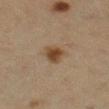The lesion was photographed on a routine skin check and not biopsied; there is no pathology result.
From the right lower leg.
Automated image analysis of the tile measured a footprint of about 6 mm², a shape eccentricity near 0.6, and two-axis asymmetry of about 0.25. The analysis additionally found an average lesion color of about L≈38 a*≈14 b*≈27 (CIELAB), about 9 CIELAB-L* units darker than the surrounding skin, and a lesion-to-skin contrast of about 8.5 (normalized; higher = more distinct). The analysis additionally found a border-irregularity rating of about 2/10, a within-lesion color-variation index near 3/10, and peripheral color asymmetry of about 0.5.
A female patient in their 40s.
Cropped from a total-body skin-imaging series; the visible field is about 15 mm.
Longest diameter approximately 3 mm.
The tile uses cross-polarized illumination.